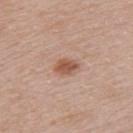| key | value |
|---|---|
| workup | imaged on a skin check; not biopsied |
| image | 15 mm crop, total-body photography |
| body site | the left upper arm |
| subject | female, about 30 years old |